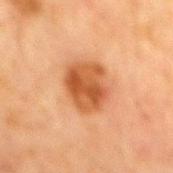| key | value |
|---|---|
| biopsy status | imaged on a skin check; not biopsied |
| body site | the mid back |
| subject | male, about 65 years old |
| TBP lesion metrics | a footprint of about 15 mm², a shape eccentricity near 0.65, and two-axis asymmetry of about 0.15; about 12 CIELAB-L* units darker than the surrounding skin; a border-irregularity rating of about 1.5/10, a within-lesion color-variation index near 5/10, and radial color variation of about 2; an automated nevus-likeness rating near 100 out of 100 and a detector confidence of about 100 out of 100 that the crop contains a lesion |
| size | ~5 mm (longest diameter) |
| image source | 15 mm crop, total-body photography |